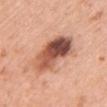Part of a total-body skin-imaging series; this lesion was reviewed on a skin check and was not flagged for biopsy. The patient is a female about 60 years old. The lesion is on the left upper arm. The lesion's longest dimension is about 6.5 mm. Cropped from a whole-body photographic skin survey; the tile spans about 15 mm.